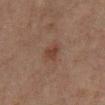Assessment: The lesion was photographed on a routine skin check and not biopsied; there is no pathology result. Acquisition and patient details: A lesion tile, about 15 mm wide, cut from a 3D total-body photograph. A female subject about 55 years old. From the leg. The lesion-visualizer software estimated an average lesion color of about L≈35 a*≈18 b*≈24 (CIELAB), roughly 7 lightness units darker than nearby skin, and a lesion-to-skin contrast of about 7 (normalized; higher = more distinct). It also reported internal color variation of about 1.5 on a 0–10 scale and radial color variation of about 0.5. The software also gave a classifier nevus-likeness of about 65/100 and a lesion-detection confidence of about 100/100. The recorded lesion diameter is about 2.5 mm.